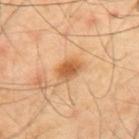Recorded during total-body skin imaging; not selected for excision or biopsy.
Approximately 3 mm at its widest.
A 15 mm close-up extracted from a 3D total-body photography capture.
Automated image analysis of the tile measured a lesion–skin lightness drop of about 12 and a normalized lesion–skin contrast near 8.5. The software also gave a border-irregularity rating of about 2/10, a color-variation rating of about 4/10, and radial color variation of about 1. The software also gave a classifier nevus-likeness of about 90/100 and a detector confidence of about 100 out of 100 that the crop contains a lesion.
Located on the mid back.
A male subject, roughly 40 years of age.